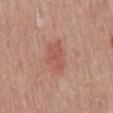patient = male, about 50 years old; illumination = white-light illumination; anatomic site = the mid back; lesion size = about 4 mm; acquisition = total-body-photography crop, ~15 mm field of view.